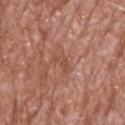Q: Is there a histopathology result?
A: catalogued during a skin exam; not biopsied
Q: What lighting was used for the tile?
A: white-light
Q: What is the lesion's diameter?
A: ≈3.5 mm
Q: Lesion location?
A: the upper back
Q: What is the imaging modality?
A: ~15 mm crop, total-body skin-cancer survey
Q: Patient demographics?
A: male, aged approximately 70